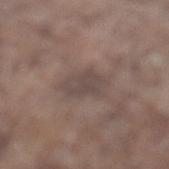Imaged during a routine full-body skin examination; the lesion was not biopsied and no histopathology is available. The lesion is on the left lower leg. A 15 mm close-up extracted from a 3D total-body photography capture. Automated image analysis of the tile measured border irregularity of about 2.5 on a 0–10 scale, a color-variation rating of about 2/10, and peripheral color asymmetry of about 0.5. And it measured an automated nevus-likeness rating near 0 out of 100 and a lesion-detection confidence of about 70/100. A male subject aged 78 to 82.